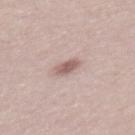Impression: Recorded during total-body skin imaging; not selected for excision or biopsy. Context: About 2.5 mm across. A lesion tile, about 15 mm wide, cut from a 3D total-body photograph. A male patient, aged 28 to 32. Captured under white-light illumination.